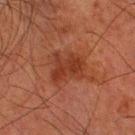| field | value |
|---|---|
| patient | male, aged 78 to 82 |
| anatomic site | the leg |
| acquisition | 15 mm crop, total-body photography |